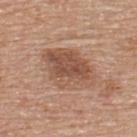This lesion was catalogued during total-body skin photography and was not selected for biopsy.
A 15 mm crop from a total-body photograph taken for skin-cancer surveillance.
Located on the upper back.
A male subject in their mid- to late 50s.
Measured at roughly 6.5 mm in maximum diameter.